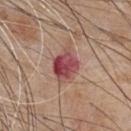Clinical impression:
Imaged during a routine full-body skin examination; the lesion was not biopsied and no histopathology is available.